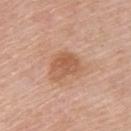No biopsy was performed on this lesion — it was imaged during a full skin examination and was not determined to be concerning.
The lesion is on the upper back.
Approximately 4 mm at its widest.
Captured under white-light illumination.
Cropped from a whole-body photographic skin survey; the tile spans about 15 mm.
A female subject about 65 years old.